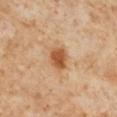Findings:
• biopsy status — no biopsy performed (imaged during a skin exam)
• tile lighting — cross-polarized illumination
• image source — ~15 mm crop, total-body skin-cancer survey
• diameter — ~3 mm (longest diameter)
• location — the right lower leg
• image-analysis metrics — an average lesion color of about L≈55 a*≈24 b*≈39 (CIELAB), about 14 CIELAB-L* units darker than the surrounding skin, and a normalized lesion–skin contrast near 9.5; a lesion-detection confidence of about 100/100
• subject — female, in their 50s